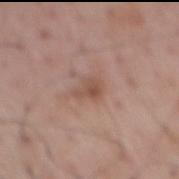Located on the mid back. The tile uses white-light illumination. Cropped from a total-body skin-imaging series; the visible field is about 15 mm. The patient is a male aged around 70. An algorithmic analysis of the crop reported a footprint of about 4.5 mm², a shape eccentricity near 0.7, and two-axis asymmetry of about 0.35. And it measured border irregularity of about 3 on a 0–10 scale, internal color variation of about 3.5 on a 0–10 scale, and peripheral color asymmetry of about 1.5.